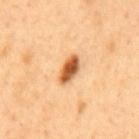{"biopsy_status": "not biopsied; imaged during a skin examination", "patient": {"sex": "male", "age_approx": 50}, "image": {"source": "total-body photography crop", "field_of_view_mm": 15}, "automated_metrics": {"border_irregularity_0_10": 2.0, "color_variation_0_10": 5.0, "peripheral_color_asymmetry": 1.5}, "site": "mid back"}